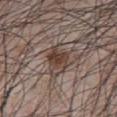image-analysis metrics: a lesion color around L≈40 a*≈15 b*≈22 in CIELAB and a lesion-to-skin contrast of about 9 (normalized; higher = more distinct); border irregularity of about 3.5 on a 0–10 scale, a color-variation rating of about 4/10, and radial color variation of about 1.5; an automated nevus-likeness rating near 95 out of 100 and a detector confidence of about 100 out of 100 that the crop contains a lesion
subject: male, aged 68 to 72
location: the front of the torso
tile lighting: white-light illumination
image source: ~15 mm tile from a whole-body skin photo
lesion size: about 3 mm